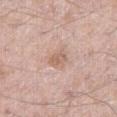notes — catalogued during a skin exam; not biopsied
lighting — white-light illumination
image-analysis metrics — a lesion area of about 4 mm²; a lesion–skin lightness drop of about 8 and a normalized border contrast of about 6; border irregularity of about 1.5 on a 0–10 scale and internal color variation of about 3 on a 0–10 scale; a classifier nevus-likeness of about 0/100 and a lesion-detection confidence of about 100/100
site — the right thigh
subject — male, about 65 years old
image source — total-body-photography crop, ~15 mm field of view
size — ≈2.5 mm